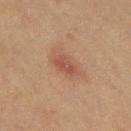Recorded during total-body skin imaging; not selected for excision or biopsy.
A 15 mm close-up tile from a total-body photography series done for melanoma screening.
A female patient approximately 50 years of age.
Measured at roughly 3 mm in maximum diameter.
This is a cross-polarized tile.
The lesion is located on the left thigh.